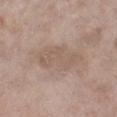– workup — no biopsy performed (imaged during a skin exam)
– subject — female, about 75 years old
– size — ≈6 mm
– imaging modality — ~15 mm tile from a whole-body skin photo
– automated lesion analysis — a footprint of about 15 mm² and an eccentricity of roughly 0.85; a border-irregularity index near 3/10, internal color variation of about 2 on a 0–10 scale, and peripheral color asymmetry of about 0.5; an automated nevus-likeness rating near 0 out of 100 and a lesion-detection confidence of about 100/100
– location — the right lower leg